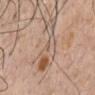{"biopsy_status": "not biopsied; imaged during a skin examination", "patient": {"sex": "male", "age_approx": 55}, "lighting": "white-light", "site": "front of the torso", "automated_metrics": {"area_mm2_approx": 15.0, "eccentricity": 0.95, "shape_asymmetry": 0.55, "nevus_likeness_0_100": 5, "lesion_detection_confidence_0_100": 65}, "lesion_size": {"long_diameter_mm_approx": 8.0}, "image": {"source": "total-body photography crop", "field_of_view_mm": 15}}